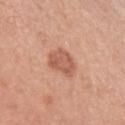- notes — no biopsy performed (imaged during a skin exam)
- patient — female, in their mid- to late 60s
- image — ~15 mm crop, total-body skin-cancer survey
- illumination — white-light illumination
- body site — the arm